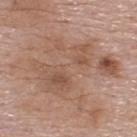Recorded during total-body skin imaging; not selected for excision or biopsy.
The recorded lesion diameter is about 11 mm.
Captured under white-light illumination.
From the upper back.
A male patient aged approximately 55.
The lesion-visualizer software estimated an area of roughly 49 mm², an eccentricity of roughly 0.7, and two-axis asymmetry of about 0.45. The software also gave a mean CIELAB color near L≈55 a*≈19 b*≈28, roughly 8 lightness units darker than nearby skin, and a normalized border contrast of about 6.
A lesion tile, about 15 mm wide, cut from a 3D total-body photograph.